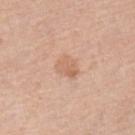Part of a total-body skin-imaging series; this lesion was reviewed on a skin check and was not flagged for biopsy. The patient is a female in their mid- to late 50s. Imaged with white-light lighting. A 15 mm close-up extracted from a 3D total-body photography capture. The lesion-visualizer software estimated a lesion area of about 5.5 mm², a shape eccentricity near 0.55, and a shape-asymmetry score of about 0.25 (0 = symmetric). The software also gave a border-irregularity rating of about 2/10, a color-variation rating of about 2.5/10, and a peripheral color-asymmetry measure near 1. The lesion is on the left lower leg. The recorded lesion diameter is about 3 mm.